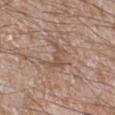The lesion was photographed on a routine skin check and not biopsied; there is no pathology result.
The lesion is located on the right lower leg.
The recorded lesion diameter is about 3 mm.
A 15 mm close-up extracted from a 3D total-body photography capture.
Imaged with white-light lighting.
A male subject in their 60s.